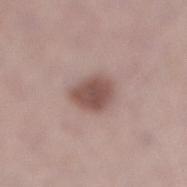Part of a total-body skin-imaging series; this lesion was reviewed on a skin check and was not flagged for biopsy. A 15 mm close-up tile from a total-body photography series done for melanoma screening. A female subject aged 48 to 52. The lesion is on the leg.